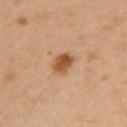biopsy status: no biopsy performed (imaged during a skin exam); imaging modality: total-body-photography crop, ~15 mm field of view; subject: female, approximately 40 years of age; body site: the arm; size: ≈3 mm; illumination: cross-polarized illumination.